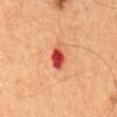patient — male, approximately 65 years of age
imaging modality — ~15 mm crop, total-body skin-cancer survey
location — the mid back
lighting — cross-polarized illumination
lesion diameter — ~3 mm (longest diameter)
image-analysis metrics — a footprint of about 4.5 mm², a shape eccentricity near 0.75, and a shape-asymmetry score of about 0.2 (0 = symmetric); a border-irregularity index near 2/10, a within-lesion color-variation index near 3/10, and peripheral color asymmetry of about 1; an automated nevus-likeness rating near 0 out of 100 and a lesion-detection confidence of about 100/100